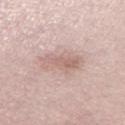{"biopsy_status": "not biopsied; imaged during a skin examination", "patient": {"sex": "female", "age_approx": 70}, "site": "left thigh", "lighting": "white-light", "lesion_size": {"long_diameter_mm_approx": 4.5}, "image": {"source": "total-body photography crop", "field_of_view_mm": 15}}